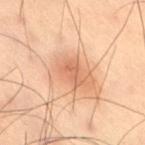No biopsy was performed on this lesion — it was imaged during a full skin examination and was not determined to be concerning.
Captured under cross-polarized illumination.
Longest diameter approximately 2.5 mm.
From the right thigh.
A male patient, in their mid- to late 50s.
A close-up tile cropped from a whole-body skin photograph, about 15 mm across.